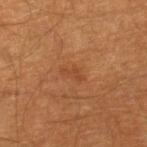Q: Was a biopsy performed?
A: catalogued during a skin exam; not biopsied
Q: What are the patient's age and sex?
A: male, aged 58 to 62
Q: What is the imaging modality?
A: ~15 mm crop, total-body skin-cancer survey
Q: Where on the body is the lesion?
A: the right lower leg
Q: How was the tile lit?
A: cross-polarized
Q: What is the lesion's diameter?
A: ≈3 mm